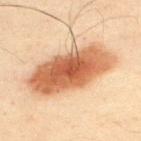Background:
A 15 mm close-up tile from a total-body photography series done for melanoma screening. A male patient aged approximately 50. The recorded lesion diameter is about 10 mm. The tile uses cross-polarized illumination. From the upper back.